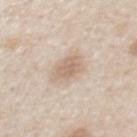{"biopsy_status": "not biopsied; imaged during a skin examination", "lighting": "white-light", "image": {"source": "total-body photography crop", "field_of_view_mm": 15}, "automated_metrics": {"cielab_L": 66, "cielab_a": 14, "cielab_b": 28, "vs_skin_contrast_norm": 6.0, "border_irregularity_0_10": 2.0, "peripheral_color_asymmetry": 0.5, "nevus_likeness_0_100": 25, "lesion_detection_confidence_0_100": 100}, "site": "chest", "lesion_size": {"long_diameter_mm_approx": 3.0}, "patient": {"sex": "male", "age_approx": 60}}